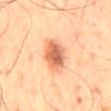Captured during whole-body skin photography for melanoma surveillance; the lesion was not biopsied.